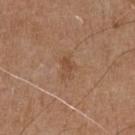<lesion>
  <biopsy_status>not biopsied; imaged during a skin examination</biopsy_status>
  <image>
    <source>total-body photography crop</source>
    <field_of_view_mm>15</field_of_view_mm>
  </image>
  <site>chest</site>
  <lighting>white-light</lighting>
  <lesion_size>
    <long_diameter_mm_approx>2.5</long_diameter_mm_approx>
  </lesion_size>
  <patient>
    <sex>male</sex>
    <age_approx>70</age_approx>
  </patient>
</lesion>